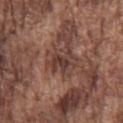Clinical impression: Part of a total-body skin-imaging series; this lesion was reviewed on a skin check and was not flagged for biopsy. Context: Measured at roughly 3 mm in maximum diameter. A roughly 15 mm field-of-view crop from a total-body skin photograph. An algorithmic analysis of the crop reported an average lesion color of about L≈38 a*≈20 b*≈23 (CIELAB) and a lesion-to-skin contrast of about 8 (normalized; higher = more distinct). A male patient, aged 73 to 77. This is a white-light tile. From the front of the torso.